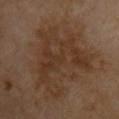Q: What are the patient's age and sex?
A: male, roughly 55 years of age
Q: Where on the body is the lesion?
A: the upper back
Q: What is the lesion's diameter?
A: ~9 mm (longest diameter)
Q: How was this image acquired?
A: ~15 mm tile from a whole-body skin photo
Q: Illumination type?
A: cross-polarized illumination